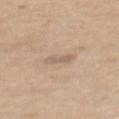Part of a total-body skin-imaging series; this lesion was reviewed on a skin check and was not flagged for biopsy.
The subject is a female in their 70s.
Imaged with white-light lighting.
A 15 mm crop from a total-body photograph taken for skin-cancer surveillance.
The lesion-visualizer software estimated a lesion area of about 3 mm², a shape eccentricity near 0.9, and two-axis asymmetry of about 0.3. The analysis additionally found a lesion color around L≈61 a*≈14 b*≈27 in CIELAB and about 8 CIELAB-L* units darker than the surrounding skin. The software also gave a classifier nevus-likeness of about 0/100.
Located on the mid back.